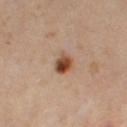Q: Was a biopsy performed?
A: no biopsy performed (imaged during a skin exam)
Q: Where on the body is the lesion?
A: the right thigh
Q: Lesion size?
A: ~2.5 mm (longest diameter)
Q: Automated lesion metrics?
A: border irregularity of about 1.5 on a 0–10 scale, a within-lesion color-variation index near 8.5/10, and peripheral color asymmetry of about 3
Q: What is the imaging modality?
A: ~15 mm tile from a whole-body skin photo
Q: Who is the patient?
A: female, roughly 40 years of age